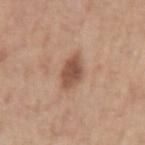No biopsy was performed on this lesion — it was imaged during a full skin examination and was not determined to be concerning.
The patient is a male roughly 75 years of age.
Captured under white-light illumination.
The lesion is located on the left upper arm.
Automated tile analysis of the lesion measured a lesion area of about 7 mm², an outline eccentricity of about 0.7 (0 = round, 1 = elongated), and a symmetry-axis asymmetry near 0.2. And it measured an average lesion color of about L≈52 a*≈21 b*≈29 (CIELAB), roughly 12 lightness units darker than nearby skin, and a normalized border contrast of about 8.5. The analysis additionally found a within-lesion color-variation index near 3/10 and a peripheral color-asymmetry measure near 1. It also reported an automated nevus-likeness rating near 60 out of 100 and lesion-presence confidence of about 100/100.
This image is a 15 mm lesion crop taken from a total-body photograph.